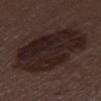Captured during whole-body skin photography for melanoma surveillance; the lesion was not biopsied.
On the leg.
A female subject about 50 years old.
This image is a 15 mm lesion crop taken from a total-body photograph.